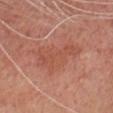Q: Is there a histopathology result?
A: catalogued during a skin exam; not biopsied
Q: What is the anatomic site?
A: the head or neck
Q: What are the patient's age and sex?
A: male, aged approximately 70
Q: How large is the lesion?
A: ~7 mm (longest diameter)
Q: How was this image acquired?
A: total-body-photography crop, ~15 mm field of view
Q: What did automated image analysis measure?
A: border irregularity of about 6 on a 0–10 scale and a within-lesion color-variation index near 2/10
Q: How was the tile lit?
A: cross-polarized